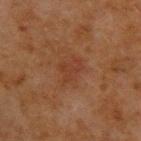notes — catalogued during a skin exam; not biopsied
site — the upper back
lesion diameter — ≈3.5 mm
automated lesion analysis — an eccentricity of roughly 0.7; a lesion–skin lightness drop of about 5; border irregularity of about 5 on a 0–10 scale and a within-lesion color-variation index near 2/10
tile lighting — cross-polarized illumination
image — 15 mm crop, total-body photography
patient — male, aged approximately 50